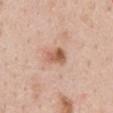Imaged during a routine full-body skin examination; the lesion was not biopsied and no histopathology is available. Longest diameter approximately 2.5 mm. The patient is a female in their mid- to late 40s. A 15 mm crop from a total-body photograph taken for skin-cancer surveillance. Captured under white-light illumination. On the abdomen. An algorithmic analysis of the crop reported a border-irregularity index near 2/10, a within-lesion color-variation index near 5.5/10, and radial color variation of about 2. It also reported a classifier nevus-likeness of about 70/100 and a detector confidence of about 100 out of 100 that the crop contains a lesion.